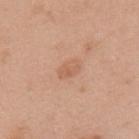Part of a total-body skin-imaging series; this lesion was reviewed on a skin check and was not flagged for biopsy. On the back. Measured at roughly 3 mm in maximum diameter. A female patient, about 50 years old. A 15 mm crop from a total-body photograph taken for skin-cancer surveillance.